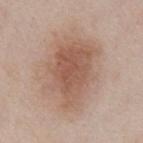{"biopsy_status": "not biopsied; imaged during a skin examination", "image": {"source": "total-body photography crop", "field_of_view_mm": 15}, "patient": {"sex": "male", "age_approx": 55}, "lesion_size": {"long_diameter_mm_approx": 8.0}, "automated_metrics": {"color_variation_0_10": 4.0, "peripheral_color_asymmetry": 1.0, "lesion_detection_confidence_0_100": 100}, "site": "chest", "lighting": "white-light"}